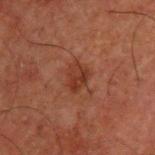Q: Was this lesion biopsied?
A: imaged on a skin check; not biopsied
Q: What lighting was used for the tile?
A: cross-polarized illumination
Q: Who is the patient?
A: male, in their 50s
Q: What is the imaging modality?
A: ~15 mm crop, total-body skin-cancer survey
Q: Where on the body is the lesion?
A: the upper back
Q: What is the lesion's diameter?
A: about 3 mm